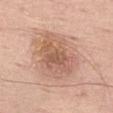Captured during whole-body skin photography for melanoma surveillance; the lesion was not biopsied.
The lesion-visualizer software estimated a mean CIELAB color near L≈60 a*≈21 b*≈30, a lesion–skin lightness drop of about 10, and a normalized border contrast of about 6.5. The software also gave border irregularity of about 3 on a 0–10 scale, internal color variation of about 5.5 on a 0–10 scale, and peripheral color asymmetry of about 1.5.
A male subject, aged around 75.
The recorded lesion diameter is about 7 mm.
A close-up tile cropped from a whole-body skin photograph, about 15 mm across.
On the left thigh.
The tile uses white-light illumination.